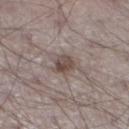Case summary:
• acquisition — total-body-photography crop, ~15 mm field of view
• location — the left lower leg
• subject — male, in their 70s